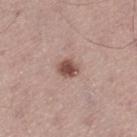The tile uses white-light illumination. An algorithmic analysis of the crop reported an area of roughly 4.5 mm² and a symmetry-axis asymmetry near 0.25. The software also gave a nevus-likeness score of about 90/100 and lesion-presence confidence of about 100/100. A 15 mm close-up tile from a total-body photography series done for melanoma screening. A male subject roughly 55 years of age. Located on the left thigh. About 2.5 mm across.